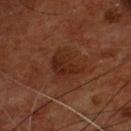The lesion was tiled from a total-body skin photograph and was not biopsied. The tile uses cross-polarized illumination. Located on the chest. Approximately 4 mm at its widest. A close-up tile cropped from a whole-body skin photograph, about 15 mm across. A male subject, in their mid- to late 50s.